The patient is a male aged around 80. A roughly 15 mm field-of-view crop from a total-body skin photograph. On the chest — 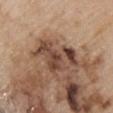* histopathologic diagnosis · a melanoma in situ (malignant)